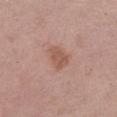{
  "biopsy_status": "not biopsied; imaged during a skin examination",
  "site": "leg",
  "image": {
    "source": "total-body photography crop",
    "field_of_view_mm": 15
  },
  "patient": {
    "sex": "female",
    "age_approx": 60
  }
}